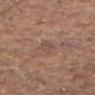biopsy status: catalogued during a skin exam; not biopsied
acquisition: total-body-photography crop, ~15 mm field of view
size: ≈2.5 mm
anatomic site: the chest
patient: male, aged 78–82
tile lighting: white-light illumination